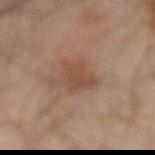Assessment:
No biopsy was performed on this lesion — it was imaged during a full skin examination and was not determined to be concerning.
Background:
Longest diameter approximately 4 mm. A 15 mm crop from a total-body photograph taken for skin-cancer surveillance. A male patient aged 58–62. The tile uses cross-polarized illumination. Located on the abdomen.